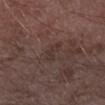* biopsy status · imaged on a skin check; not biopsied
* acquisition · 15 mm crop, total-body photography
* diameter · ≈3 mm
* subject · male, in their mid- to late 70s
* location · the right forearm
* lighting · white-light